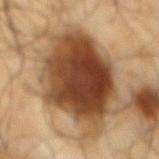Clinical summary: The lesion is on the mid back. A male subject, aged 63–67. Approximately 11.5 mm at its widest. Cropped from a total-body skin-imaging series; the visible field is about 15 mm.